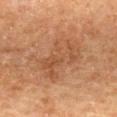{"patient": {"sex": "female", "age_approx": 60}, "lesion_size": {"long_diameter_mm_approx": 6.5}, "lighting": "cross-polarized", "site": "back", "image": {"source": "total-body photography crop", "field_of_view_mm": 15}}